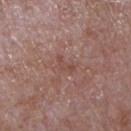follow-up: no biopsy performed (imaged during a skin exam) | diameter: ≈2.5 mm | anatomic site: the chest | illumination: white-light illumination | image source: total-body-photography crop, ~15 mm field of view | patient: male, approximately 65 years of age | automated metrics: an area of roughly 2.5 mm², an outline eccentricity of about 0.9 (0 = round, 1 = elongated), and a shape-asymmetry score of about 0.55 (0 = symmetric); a lesion color around L≈47 a*≈21 b*≈24 in CIELAB, about 6 CIELAB-L* units darker than the surrounding skin, and a lesion-to-skin contrast of about 5 (normalized; higher = more distinct); an automated nevus-likeness rating near 0 out of 100 and a detector confidence of about 100 out of 100 that the crop contains a lesion.